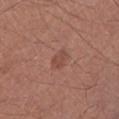Part of a total-body skin-imaging series; this lesion was reviewed on a skin check and was not flagged for biopsy. The lesion is located on the left lower leg. A region of skin cropped from a whole-body photographic capture, roughly 15 mm wide. The patient is a male aged 68–72.